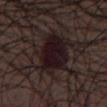Part of a total-body skin-imaging series; this lesion was reviewed on a skin check and was not flagged for biopsy. A male subject, approximately 50 years of age. A 15 mm close-up extracted from a 3D total-body photography capture.